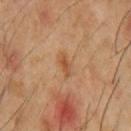{"biopsy_status": "not biopsied; imaged during a skin examination", "lesion_size": {"long_diameter_mm_approx": 2.5}, "site": "front of the torso", "image": {"source": "total-body photography crop", "field_of_view_mm": 15}, "lighting": "cross-polarized", "patient": {"sex": "male", "age_approx": 60}}